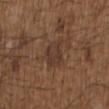This lesion was catalogued during total-body skin photography and was not selected for biopsy. The subject is a male aged 48 to 52. This is a white-light tile. Cropped from a whole-body photographic skin survey; the tile spans about 15 mm. On the upper back. Longest diameter approximately 3 mm.